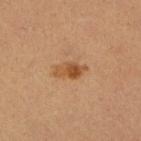biopsy_status: not biopsied; imaged during a skin examination
lesion_size:
  long_diameter_mm_approx: 3.5
automated_metrics:
  shape_asymmetry: 0.3
  vs_skin_darker_L: 11.0
  vs_skin_contrast_norm: 8.5
  nevus_likeness_0_100: 85
  lesion_detection_confidence_0_100: 100
patient:
  sex: female
  age_approx: 35
lighting: cross-polarized
image:
  source: total-body photography crop
  field_of_view_mm: 15
site: right lower leg